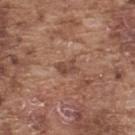No biopsy was performed on this lesion — it was imaged during a full skin examination and was not determined to be concerning.
A male subject aged 73 to 77.
Longest diameter approximately 2.5 mm.
A lesion tile, about 15 mm wide, cut from a 3D total-body photograph.
The lesion is on the back.